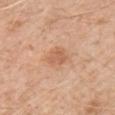Automated image analysis of the tile measured a mean CIELAB color near L≈60 a*≈23 b*≈35.
The tile uses white-light illumination.
A roughly 15 mm field-of-view crop from a total-body skin photograph.
Approximately 2.5 mm at its widest.
A male patient aged 58–62.
The lesion is on the right upper arm.